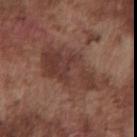| key | value |
|---|---|
| follow-up | no biopsy performed (imaged during a skin exam) |
| illumination | white-light illumination |
| image-analysis metrics | a footprint of about 22 mm², an outline eccentricity of about 0.9 (0 = round, 1 = elongated), and two-axis asymmetry of about 0.3 |
| location | the front of the torso |
| image | ~15 mm crop, total-body skin-cancer survey |
| patient | male, in their mid-70s |
| lesion diameter | ≈8.5 mm |